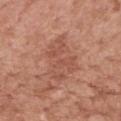follow-up: total-body-photography surveillance lesion; no biopsy | acquisition: total-body-photography crop, ~15 mm field of view | site: the mid back | illumination: white-light illumination | subject: male, in their 70s | size: ≈5 mm.